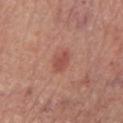The lesion was tiled from a total-body skin photograph and was not biopsied. Captured under white-light illumination. A male patient, roughly 70 years of age. The lesion is on the left lower leg. A 15 mm close-up extracted from a 3D total-body photography capture.